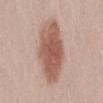Case summary:
• notes — catalogued during a skin exam; not biopsied
• image source — ~15 mm tile from a whole-body skin photo
• body site — the back
• tile lighting — white-light illumination
• patient — male, in their mid- to late 20s
• TBP lesion metrics — a lesion area of about 27 mm² and a symmetry-axis asymmetry near 0.1; a mean CIELAB color near L≈57 a*≈20 b*≈27 and a normalized border contrast of about 9